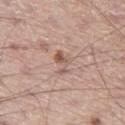No biopsy was performed on this lesion — it was imaged during a full skin examination and was not determined to be concerning. A lesion tile, about 15 mm wide, cut from a 3D total-body photograph. This is a white-light tile. The patient is a male approximately 65 years of age. The lesion is located on the left thigh.